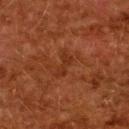Q: Was a biopsy performed?
A: no biopsy performed (imaged during a skin exam)
Q: What are the patient's age and sex?
A: female, about 50 years old
Q: How was this image acquired?
A: ~15 mm tile from a whole-body skin photo
Q: How large is the lesion?
A: about 3 mm
Q: Illumination type?
A: cross-polarized illumination
Q: Automated lesion metrics?
A: an eccentricity of roughly 0.9; a mean CIELAB color near L≈26 a*≈23 b*≈29, roughly 5 lightness units darker than nearby skin, and a normalized lesion–skin contrast near 5.5; border irregularity of about 4 on a 0–10 scale and a peripheral color-asymmetry measure near 0
Q: Lesion location?
A: the upper back